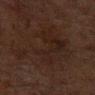Clinical summary:
Located on the left forearm. About 9.5 mm across. The subject is a female roughly 50 years of age. A region of skin cropped from a whole-body photographic capture, roughly 15 mm wide.